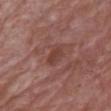No biopsy was performed on this lesion — it was imaged during a full skin examination and was not determined to be concerning. The total-body-photography lesion software estimated a lesion area of about 4 mm², an outline eccentricity of about 0.75 (0 = round, 1 = elongated), and two-axis asymmetry of about 0.15. It also reported a border-irregularity index near 1/10, a within-lesion color-variation index near 2/10, and a peripheral color-asymmetry measure near 0.5. It also reported a classifier nevus-likeness of about 0/100 and lesion-presence confidence of about 100/100. A region of skin cropped from a whole-body photographic capture, roughly 15 mm wide. The patient is a male in their mid-80s or older. Located on the front of the torso. Imaged with white-light lighting. Longest diameter approximately 3 mm.